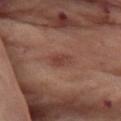{"biopsy_status": "not biopsied; imaged during a skin examination", "lesion_size": {"long_diameter_mm_approx": 2.5}, "site": "abdomen", "automated_metrics": {"vs_skin_darker_L": 8.0, "nevus_likeness_0_100": 85, "lesion_detection_confidence_0_100": 100}, "patient": {"sex": "female", "age_approx": 55}, "image": {"source": "total-body photography crop", "field_of_view_mm": 15}}